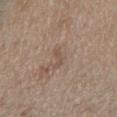Q: Was this lesion biopsied?
A: total-body-photography surveillance lesion; no biopsy
Q: What is the lesion's diameter?
A: ~3 mm (longest diameter)
Q: Lesion location?
A: the right upper arm
Q: What are the patient's age and sex?
A: male, roughly 55 years of age
Q: What lighting was used for the tile?
A: white-light
Q: What kind of image is this?
A: ~15 mm crop, total-body skin-cancer survey